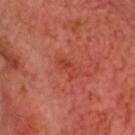Part of a total-body skin-imaging series; this lesion was reviewed on a skin check and was not flagged for biopsy.
The patient is a male aged around 65.
This is a cross-polarized tile.
A 15 mm crop from a total-body photograph taken for skin-cancer surveillance.